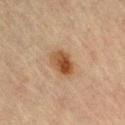{
  "biopsy_status": "not biopsied; imaged during a skin examination",
  "image": {
    "source": "total-body photography crop",
    "field_of_view_mm": 15
  },
  "site": "leg",
  "patient": {
    "sex": "female",
    "age_approx": 65
  }
}